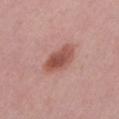Case summary:
– workup · imaged on a skin check; not biopsied